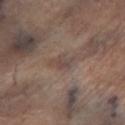follow-up = no biopsy performed (imaged during a skin exam)
automated lesion analysis = border irregularity of about 4 on a 0–10 scale and a within-lesion color-variation index near 1.5/10; a nevus-likeness score of about 0/100
anatomic site = the left lower leg
lesion size = ≈3 mm
acquisition = 15 mm crop, total-body photography
lighting = cross-polarized illumination